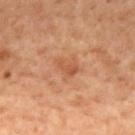Clinical impression: The lesion was photographed on a routine skin check and not biopsied; there is no pathology result. Clinical summary: An algorithmic analysis of the crop reported a footprint of about 5 mm², an outline eccentricity of about 0.85 (0 = round, 1 = elongated), and a symmetry-axis asymmetry near 0.35. And it measured a nevus-likeness score of about 0/100 and a detector confidence of about 100 out of 100 that the crop contains a lesion. A close-up tile cropped from a whole-body skin photograph, about 15 mm across. From the mid back. The recorded lesion diameter is about 3.5 mm. This is a cross-polarized tile. A male patient, in their 70s.